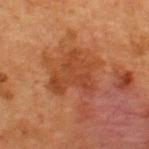Q: Was this lesion biopsied?
A: no biopsy performed (imaged during a skin exam)
Q: What is the imaging modality?
A: ~15 mm crop, total-body skin-cancer survey
Q: What are the patient's age and sex?
A: female, in their 40s
Q: What is the lesion's diameter?
A: ~5 mm (longest diameter)
Q: What did automated image analysis measure?
A: a classifier nevus-likeness of about 0/100
Q: Illumination type?
A: cross-polarized
Q: Where on the body is the lesion?
A: the upper back